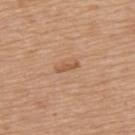<case>
<image>
  <source>total-body photography crop</source>
  <field_of_view_mm>15</field_of_view_mm>
</image>
<lighting>white-light</lighting>
<patient>
  <sex>male</sex>
  <age_approx>65</age_approx>
</patient>
<lesion_size>
  <long_diameter_mm_approx>2.5</long_diameter_mm_approx>
</lesion_size>
<site>upper back</site>
<automated_metrics>
  <area_mm2_approx>2.0</area_mm2_approx>
  <eccentricity>0.95</eccentricity>
  <shape_asymmetry>0.3</shape_asymmetry>
  <cielab_L>55</cielab_L>
  <cielab_a>21</cielab_a>
  <cielab_b>34</cielab_b>
  <vs_skin_darker_L>9.0</vs_skin_darker_L>
  <vs_skin_contrast_norm>6.5</vs_skin_contrast_norm>
  <peripheral_color_asymmetry>0.0</peripheral_color_asymmetry>
</automated_metrics>
</case>